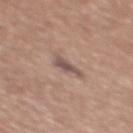workup = no biopsy performed (imaged during a skin exam) | size = ≈3 mm | automated metrics = an area of roughly 4 mm²; a mean CIELAB color near L≈52 a*≈17 b*≈21, about 10 CIELAB-L* units darker than the surrounding skin, and a normalized lesion–skin contrast near 8; a nevus-likeness score of about 0/100 and a detector confidence of about 100 out of 100 that the crop contains a lesion | image source = 15 mm crop, total-body photography | site = the upper back | patient = female, approximately 50 years of age | illumination = white-light illumination.